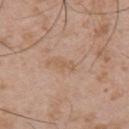biopsy_status: not biopsied; imaged during a skin examination
automated_metrics:
  cielab_L: 58
  cielab_a: 18
  cielab_b: 32
  vs_skin_darker_L: 6.0
  vs_skin_contrast_norm: 5.0
  border_irregularity_0_10: 4.5
  color_variation_0_10: 0.0
  peripheral_color_asymmetry: 0.0
  nevus_likeness_0_100: 0
site: upper back
lighting: white-light
patient:
  sex: male
  age_approx: 55
image:
  source: total-body photography crop
  field_of_view_mm: 15
lesion_size:
  long_diameter_mm_approx: 2.5